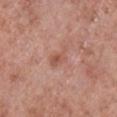Impression:
The lesion was photographed on a routine skin check and not biopsied; there is no pathology result.
Context:
A region of skin cropped from a whole-body photographic capture, roughly 15 mm wide. Longest diameter approximately 3 mm. The total-body-photography lesion software estimated a lesion area of about 4 mm² and an outline eccentricity of about 0.85 (0 = round, 1 = elongated). And it measured a mean CIELAB color near L≈54 a*≈23 b*≈29. It also reported border irregularity of about 4.5 on a 0–10 scale. Located on the right lower leg. A male patient in their mid- to late 50s.